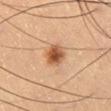Q: Was this lesion biopsied?
A: no biopsy performed (imaged during a skin exam)
Q: What are the patient's age and sex?
A: male, about 65 years old
Q: What lighting was used for the tile?
A: cross-polarized
Q: What is the lesion's diameter?
A: ≈3 mm
Q: What did automated image analysis measure?
A: a lesion color around L≈54 a*≈23 b*≈37 in CIELAB, roughly 15 lightness units darker than nearby skin, and a normalized lesion–skin contrast near 10
Q: How was this image acquired?
A: ~15 mm tile from a whole-body skin photo
Q: Lesion location?
A: the leg